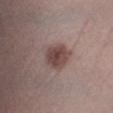workup: no biopsy performed (imaged during a skin exam); body site: the right lower leg; subject: male, in their mid- to late 40s; acquisition: 15 mm crop, total-body photography.